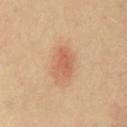<tbp_lesion>
  <biopsy_status>not biopsied; imaged during a skin examination</biopsy_status>
  <site>mid back</site>
  <patient>
    <sex>female</sex>
    <age_approx>40</age_approx>
  </patient>
  <image>
    <source>total-body photography crop</source>
    <field_of_view_mm>15</field_of_view_mm>
  </image>
</tbp_lesion>